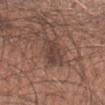Captured during whole-body skin photography for melanoma surveillance; the lesion was not biopsied.
Imaged with white-light lighting.
The total-body-photography lesion software estimated a footprint of about 8 mm², an eccentricity of roughly 0.85, and a shape-asymmetry score of about 0.25 (0 = symmetric). The analysis additionally found about 8 CIELAB-L* units darker than the surrounding skin and a lesion-to-skin contrast of about 7 (normalized; higher = more distinct). And it measured border irregularity of about 3 on a 0–10 scale, internal color variation of about 3 on a 0–10 scale, and a peripheral color-asymmetry measure near 1.
The lesion is located on the left forearm.
The lesion's longest dimension is about 4.5 mm.
The patient is a male aged around 50.
A 15 mm close-up extracted from a 3D total-body photography capture.